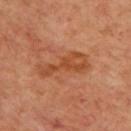Assessment:
Captured during whole-body skin photography for melanoma surveillance; the lesion was not biopsied.
Background:
A 15 mm close-up tile from a total-body photography series done for melanoma screening. Measured at roughly 6 mm in maximum diameter. The lesion is located on the upper back. The patient is a male aged approximately 65. This is a cross-polarized tile. The lesion-visualizer software estimated a lesion area of about 11 mm², an eccentricity of roughly 0.95, and a symmetry-axis asymmetry near 0.5. The software also gave an average lesion color of about L≈47 a*≈27 b*≈37 (CIELAB) and a lesion-to-skin contrast of about 7 (normalized; higher = more distinct). The analysis additionally found an automated nevus-likeness rating near 0 out of 100 and a detector confidence of about 100 out of 100 that the crop contains a lesion.